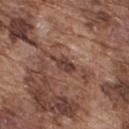Findings:
– biopsy status · imaged on a skin check; not biopsied
– subject · male, approximately 75 years of age
– automated lesion analysis · a border-irregularity rating of about 3/10, a within-lesion color-variation index near 3/10, and a peripheral color-asymmetry measure near 1
– location · the upper back
– image source · 15 mm crop, total-body photography
– lighting · white-light illumination
– lesion size · ≈3.5 mm